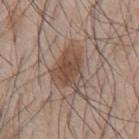Case summary:
- workup: no biopsy performed (imaged during a skin exam)
- TBP lesion metrics: an average lesion color of about L≈48 a*≈16 b*≈25 (CIELAB); a color-variation rating of about 4.5/10 and radial color variation of about 1.5
- body site: the back
- lesion size: ≈5 mm
- acquisition: 15 mm crop, total-body photography
- subject: male, in their mid-40s
- tile lighting: white-light